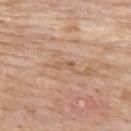Q: Is there a histopathology result?
A: no biopsy performed (imaged during a skin exam)
Q: What is the imaging modality?
A: ~15 mm crop, total-body skin-cancer survey
Q: Where on the body is the lesion?
A: the upper back
Q: Who is the patient?
A: female, approximately 65 years of age